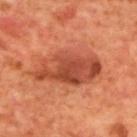The lesion was photographed on a routine skin check and not biopsied; there is no pathology result.
A male patient roughly 70 years of age.
The lesion is located on the mid back.
The lesion-visualizer software estimated a footprint of about 24 mm² and a symmetry-axis asymmetry near 0.2.
A 15 mm close-up extracted from a 3D total-body photography capture.